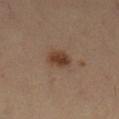Impression: The lesion was tiled from a total-body skin photograph and was not biopsied. Clinical summary: A female patient, about 45 years old. About 3 mm across. The tile uses cross-polarized illumination. A lesion tile, about 15 mm wide, cut from a 3D total-body photograph. On the left lower leg.